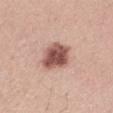Case summary:
- follow-up — total-body-photography surveillance lesion; no biopsy
- image — total-body-photography crop, ~15 mm field of view
- patient — male, aged approximately 25
- lighting — white-light illumination
- location — the upper back